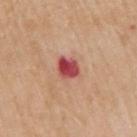Assessment: This lesion was catalogued during total-body skin photography and was not selected for biopsy. Context: The patient is a male in their mid- to late 60s. The lesion is located on the right upper arm. A lesion tile, about 15 mm wide, cut from a 3D total-body photograph. The lesion's longest dimension is about 2.5 mm.